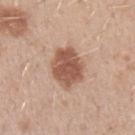Recorded during total-body skin imaging; not selected for excision or biopsy. The subject is a male about 30 years old. This image is a 15 mm lesion crop taken from a total-body photograph. An algorithmic analysis of the crop reported an outline eccentricity of about 0.65 (0 = round, 1 = elongated) and a symmetry-axis asymmetry near 0.15. It also reported a nevus-likeness score of about 85/100. From the left forearm.